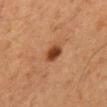{"biopsy_status": "not biopsied; imaged during a skin examination", "site": "back", "image": {"source": "total-body photography crop", "field_of_view_mm": 15}, "lighting": "cross-polarized", "patient": {"sex": "male", "age_approx": 65}, "lesion_size": {"long_diameter_mm_approx": 2.5}}